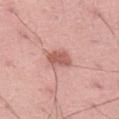| key | value |
|---|---|
| workup | imaged on a skin check; not biopsied |
| lighting | white-light |
| location | the right thigh |
| image source | ~15 mm crop, total-body skin-cancer survey |
| automated metrics | a mean CIELAB color near L≈58 a*≈26 b*≈26, a lesion–skin lightness drop of about 12, and a normalized border contrast of about 8; border irregularity of about 2 on a 0–10 scale, a within-lesion color-variation index near 2/10, and peripheral color asymmetry of about 0.5 |
| lesion diameter | ≈3 mm |
| patient | male, aged 43 to 47 |